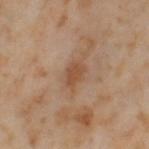The lesion was tiled from a total-body skin photograph and was not biopsied. A lesion tile, about 15 mm wide, cut from a 3D total-body photograph. A female subject in their mid- to late 50s. The lesion is located on the left thigh. Captured under cross-polarized illumination. The recorded lesion diameter is about 3.5 mm.